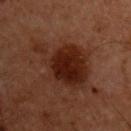  biopsy_status: not biopsied; imaged during a skin examination
  site: chest
  patient:
    sex: male
    age_approx: 60
  lighting: cross-polarized
  lesion_size:
    long_diameter_mm_approx: 7.0
  image:
    source: total-body photography crop
    field_of_view_mm: 15